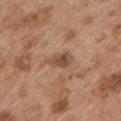anatomic site: the left upper arm; imaging modality: total-body-photography crop, ~15 mm field of view; subject: male, aged around 55; lesion diameter: ~3.5 mm (longest diameter).